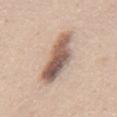The lesion was photographed on a routine skin check and not biopsied; there is no pathology result.